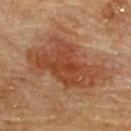| key | value |
|---|---|
| follow-up | catalogued during a skin exam; not biopsied |
| patient | male, aged approximately 85 or older |
| size | ≈9.5 mm |
| location | the back |
| acquisition | total-body-photography crop, ~15 mm field of view |
| tile lighting | cross-polarized |